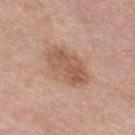No biopsy was performed on this lesion — it was imaged during a full skin examination and was not determined to be concerning.
From the right thigh.
About 5.5 mm across.
This image is a 15 mm lesion crop taken from a total-body photograph.
Captured under white-light illumination.
The total-body-photography lesion software estimated a footprint of about 12 mm² and two-axis asymmetry of about 0.2. The analysis additionally found a lesion–skin lightness drop of about 10 and a lesion-to-skin contrast of about 7 (normalized; higher = more distinct). It also reported border irregularity of about 2.5 on a 0–10 scale and a color-variation rating of about 4/10. The software also gave an automated nevus-likeness rating near 30 out of 100.
A male subject, aged 73–77.